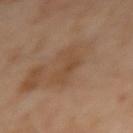<tbp_lesion>
  <biopsy_status>not biopsied; imaged during a skin examination</biopsy_status>
  <image>
    <source>total-body photography crop</source>
    <field_of_view_mm>15</field_of_view_mm>
  </image>
  <lighting>cross-polarized</lighting>
  <patient>
    <sex>female</sex>
    <age_approx>55</age_approx>
  </patient>
  <site>right upper arm</site>
  <lesion_size>
    <long_diameter_mm_approx>4.5</long_diameter_mm_approx>
  </lesion_size>
</tbp_lesion>